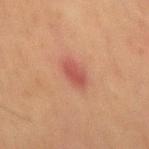No biopsy was performed on this lesion — it was imaged during a full skin examination and was not determined to be concerning. Automated tile analysis of the lesion measured a lesion–skin lightness drop of about 8 and a lesion-to-skin contrast of about 7 (normalized; higher = more distinct). A lesion tile, about 15 mm wide, cut from a 3D total-body photograph. Located on the abdomen. The recorded lesion diameter is about 3 mm. A male subject approximately 70 years of age.